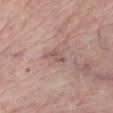Clinical impression:
Part of a total-body skin-imaging series; this lesion was reviewed on a skin check and was not flagged for biopsy.
Image and clinical context:
A close-up tile cropped from a whole-body skin photograph, about 15 mm across. Located on the chest. A male patient, aged 78–82. Imaged with white-light lighting. The lesion-visualizer software estimated an area of roughly 3.5 mm², an eccentricity of roughly 0.9, and two-axis asymmetry of about 0.45. The analysis additionally found a lesion-to-skin contrast of about 6 (normalized; higher = more distinct). And it measured border irregularity of about 4.5 on a 0–10 scale, a within-lesion color-variation index near 2.5/10, and radial color variation of about 0.5.